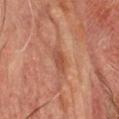The lesion was tiled from a total-body skin photograph and was not biopsied.
A male subject, in their mid- to late 70s.
Cropped from a whole-body photographic skin survey; the tile spans about 15 mm.
The lesion is on the head or neck.
This is a cross-polarized tile.